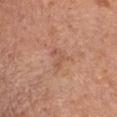The lesion was tiled from a total-body skin photograph and was not biopsied. Cropped from a total-body skin-imaging series; the visible field is about 15 mm. The patient is a female about 55 years old. The lesion is located on the chest.